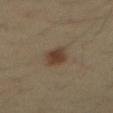Notes:
- workup: imaged on a skin check; not biopsied
- acquisition: total-body-photography crop, ~15 mm field of view
- site: the abdomen
- lighting: cross-polarized illumination
- lesion diameter: ≈3.5 mm
- patient: male, aged 33 to 37